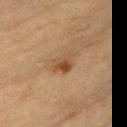Notes:
- notes — no biopsy performed (imaged during a skin exam)
- automated lesion analysis — a shape eccentricity near 0.5 and a shape-asymmetry score of about 0.35 (0 = symmetric); a nevus-likeness score of about 65/100
- image source — ~15 mm tile from a whole-body skin photo
- size — ~2.5 mm (longest diameter)
- subject — male, in their mid- to late 80s or older
- body site — the back
- illumination — cross-polarized illumination